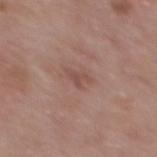Clinical summary:
Automated tile analysis of the lesion measured a footprint of about 3 mm². And it measured about 7 CIELAB-L* units darker than the surrounding skin and a normalized border contrast of about 5.5. The software also gave border irregularity of about 6 on a 0–10 scale, a color-variation rating of about 0.5/10, and peripheral color asymmetry of about 0. The software also gave an automated nevus-likeness rating near 0 out of 100 and a lesion-detection confidence of about 100/100. Imaged with white-light lighting. The lesion is on the upper back. A 15 mm close-up tile from a total-body photography series done for melanoma screening. A female subject aged 48 to 52.